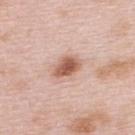Clinical impression:
The lesion was tiled from a total-body skin photograph and was not biopsied.
Background:
An algorithmic analysis of the crop reported a border-irregularity rating of about 2/10, a color-variation rating of about 3/10, and radial color variation of about 1. It also reported an automated nevus-likeness rating near 95 out of 100 and lesion-presence confidence of about 100/100. Imaged with white-light lighting. Measured at roughly 3.5 mm in maximum diameter. The lesion is located on the back. A roughly 15 mm field-of-view crop from a total-body skin photograph. The subject is a female in their mid- to late 60s.